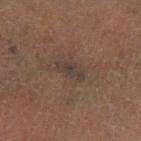biopsy_status: not biopsied; imaged during a skin examination
site: leg
automated_metrics:
  area_mm2_approx: 3.5
  eccentricity: 0.9
  shape_asymmetry: 0.3
  cielab_L: 27
  cielab_a: 9
  cielab_b: 15
  vs_skin_darker_L: 5.0
  vs_skin_contrast_norm: 7.0
  border_irregularity_0_10: 3.5
  color_variation_0_10: 1.5
  peripheral_color_asymmetry: 0.0
  nevus_likeness_0_100: 0
  lesion_detection_confidence_0_100: 65
patient:
  sex: male
  age_approx: 60
image:
  source: total-body photography crop
  field_of_view_mm: 15